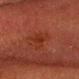Clinical impression: Imaged during a routine full-body skin examination; the lesion was not biopsied and no histopathology is available. Clinical summary: The lesion is located on the head or neck. A male subject, aged around 60. Approximately 3 mm at its widest. This is a cross-polarized tile. Cropped from a whole-body photographic skin survey; the tile spans about 15 mm.